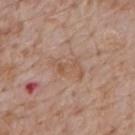Q: Was a biopsy performed?
A: total-body-photography surveillance lesion; no biopsy
Q: Where on the body is the lesion?
A: the mid back
Q: How was this image acquired?
A: 15 mm crop, total-body photography
Q: Automated lesion metrics?
A: an area of roughly 8.5 mm², an outline eccentricity of about 0.8 (0 = round, 1 = elongated), and a shape-asymmetry score of about 0.3 (0 = symmetric); border irregularity of about 3 on a 0–10 scale and internal color variation of about 4 on a 0–10 scale; an automated nevus-likeness rating near 0 out of 100 and a detector confidence of about 100 out of 100 that the crop contains a lesion
Q: What is the lesion's diameter?
A: ≈4.5 mm
Q: Illumination type?
A: white-light illumination
Q: Who is the patient?
A: male, aged 58–62